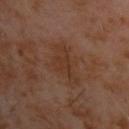follow-up: imaged on a skin check; not biopsied
patient: male, about 60 years old
image: 15 mm crop, total-body photography
body site: the upper back